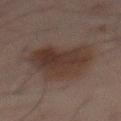This lesion was catalogued during total-body skin photography and was not selected for biopsy. Cropped from a whole-body photographic skin survey; the tile spans about 15 mm. A male patient, in their mid- to late 60s. From the mid back.